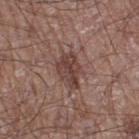The lesion was tiled from a total-body skin photograph and was not biopsied.
This is a white-light tile.
Approximately 4 mm at its widest.
Cropped from a total-body skin-imaging series; the visible field is about 15 mm.
The lesion is on the right thigh.
A male subject aged approximately 60.